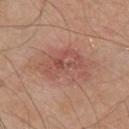Findings:
– biopsy status: catalogued during a skin exam; not biopsied
– lighting: white-light
– image-analysis metrics: a footprint of about 13 mm², a shape eccentricity near 0.8, and two-axis asymmetry of about 0.5; a mean CIELAB color near L≈52 a*≈25 b*≈26 and a lesion-to-skin contrast of about 5.5 (normalized; higher = more distinct)
– lesion diameter: ≈5.5 mm
– subject: male, roughly 80 years of age
– imaging modality: ~15 mm tile from a whole-body skin photo
– location: the front of the torso